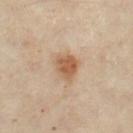{
  "biopsy_status": "not biopsied; imaged during a skin examination",
  "automated_metrics": {
    "cielab_L": 57,
    "cielab_a": 19,
    "cielab_b": 33,
    "vs_skin_darker_L": 11.0,
    "lesion_detection_confidence_0_100": 100
  },
  "patient": {
    "sex": "female",
    "age_approx": 60
  },
  "image": {
    "source": "total-body photography crop",
    "field_of_view_mm": 15
  },
  "lighting": "cross-polarized",
  "lesion_size": {
    "long_diameter_mm_approx": 3.0
  },
  "site": "left thigh"
}